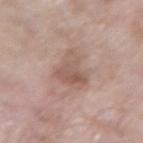biopsy status: catalogued during a skin exam; not biopsied
acquisition: ~15 mm crop, total-body skin-cancer survey
diameter: ~4 mm (longest diameter)
automated metrics: a lesion area of about 9.5 mm², a shape eccentricity near 0.7, and a shape-asymmetry score of about 0.5 (0 = symmetric); a lesion color around L≈56 a*≈18 b*≈25 in CIELAB, roughly 9 lightness units darker than nearby skin, and a lesion-to-skin contrast of about 6.5 (normalized; higher = more distinct); a border-irregularity index near 5/10 and a within-lesion color-variation index near 4/10
illumination: white-light
location: the left forearm
patient: male, in their 60s